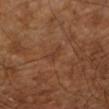patient:
  age_approx: 65
site: leg
lesion_size:
  long_diameter_mm_approx: 2.5
lighting: cross-polarized
image:
  source: total-body photography crop
  field_of_view_mm: 15